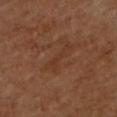The lesion was tiled from a total-body skin photograph and was not biopsied. A male subject, aged around 60. The lesion is located on the back. Automated tile analysis of the lesion measured a mean CIELAB color near L≈33 a*≈20 b*≈29 and about 5 CIELAB-L* units darker than the surrounding skin. And it measured border irregularity of about 8 on a 0–10 scale and a within-lesion color-variation index near 1/10. It also reported an automated nevus-likeness rating near 0 out of 100 and a lesion-detection confidence of about 100/100. Imaged with cross-polarized lighting. A roughly 15 mm field-of-view crop from a total-body skin photograph.